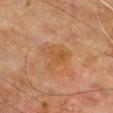image source: ~15 mm tile from a whole-body skin photo | subject: male, aged around 65 | body site: the front of the torso.